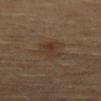workup=catalogued during a skin exam; not biopsied
imaging modality=15 mm crop, total-body photography
body site=the back
patient=female, in their 80s
automated metrics=an area of roughly 7 mm², an eccentricity of roughly 0.8, and a symmetry-axis asymmetry near 0.3; about 5 CIELAB-L* units darker than the surrounding skin and a normalized lesion–skin contrast near 5.5; border irregularity of about 3.5 on a 0–10 scale